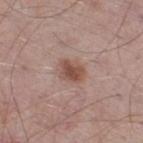notes — total-body-photography surveillance lesion; no biopsy | diameter — ≈3 mm | tile lighting — white-light | patient — male, aged approximately 55 | body site — the right thigh | image source — ~15 mm crop, total-body skin-cancer survey.